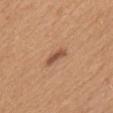{
  "automated_metrics": {
    "eccentricity": 0.95,
    "shape_asymmetry": 0.3,
    "cielab_L": 52,
    "cielab_a": 23,
    "cielab_b": 32,
    "vs_skin_contrast_norm": 8.0,
    "border_irregularity_0_10": 3.5,
    "color_variation_0_10": 0.5
  },
  "lesion_size": {
    "long_diameter_mm_approx": 3.0
  },
  "patient": {
    "sex": "female",
    "age_approx": 40
  },
  "image": {
    "source": "total-body photography crop",
    "field_of_view_mm": 15
  },
  "site": "mid back",
  "lighting": "white-light"
}